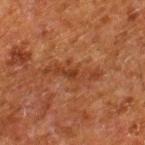location — the right lower leg
subject — male, aged 78–82
imaging modality — ~15 mm crop, total-body skin-cancer survey
lighting — cross-polarized
lesion diameter — about 6 mm